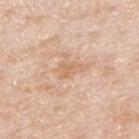body site = the right forearm
image source = 15 mm crop, total-body photography
illumination = white-light
subject = female, aged 58 to 62